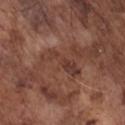{
  "biopsy_status": "not biopsied; imaged during a skin examination",
  "patient": {
    "sex": "male",
    "age_approx": 75
  },
  "lesion_size": {
    "long_diameter_mm_approx": 5.5
  },
  "lighting": "white-light",
  "image": {
    "source": "total-body photography crop",
    "field_of_view_mm": 15
  },
  "site": "chest",
  "automated_metrics": {
    "cielab_L": 38,
    "cielab_a": 21,
    "cielab_b": 25,
    "vs_skin_contrast_norm": 6.5,
    "border_irregularity_0_10": 6.5,
    "peripheral_color_asymmetry": 0.5,
    "nevus_likeness_0_100": 0,
    "lesion_detection_confidence_0_100": 65
  }
}